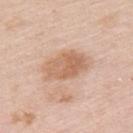lesion_size:
  long_diameter_mm_approx: 5.5
automated_metrics:
  area_mm2_approx: 14.0
  eccentricity: 0.8
  shape_asymmetry: 0.15
  border_irregularity_0_10: 2.0
  color_variation_0_10: 4.0
  peripheral_color_asymmetry: 1.5
  nevus_likeness_0_100: 25
site: left upper arm
lighting: white-light
patient:
  sex: female
  age_approx: 65
image:
  source: total-body photography crop
  field_of_view_mm: 15